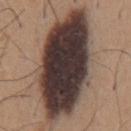Captured during whole-body skin photography for melanoma surveillance; the lesion was not biopsied. A region of skin cropped from a whole-body photographic capture, roughly 15 mm wide. An algorithmic analysis of the crop reported a mean CIELAB color near L≈36 a*≈14 b*≈18, roughly 23 lightness units darker than nearby skin, and a normalized border contrast of about 18.5. And it measured border irregularity of about 2.5 on a 0–10 scale and a color-variation rating of about 8.5/10. From the front of the torso. A male subject in their mid- to late 60s. The tile uses white-light illumination. Measured at roughly 13.5 mm in maximum diameter.